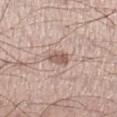Clinical impression:
The lesion was photographed on a routine skin check and not biopsied; there is no pathology result.
Context:
A male patient, aged approximately 35. The lesion's longest dimension is about 2.5 mm. A close-up tile cropped from a whole-body skin photograph, about 15 mm across. The lesion-visualizer software estimated an area of roughly 4.5 mm² and an eccentricity of roughly 0.7. And it measured a classifier nevus-likeness of about 10/100 and lesion-presence confidence of about 100/100. Imaged with white-light lighting. On the leg.